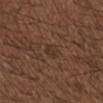Impression: This lesion was catalogued during total-body skin photography and was not selected for biopsy. Image and clinical context: Located on the back. A region of skin cropped from a whole-body photographic capture, roughly 15 mm wide. This is a white-light tile. A male patient, aged approximately 50. Measured at roughly 2.5 mm in maximum diameter. The lesion-visualizer software estimated a lesion color around L≈33 a*≈17 b*≈25 in CIELAB, about 5 CIELAB-L* units darker than the surrounding skin, and a lesion-to-skin contrast of about 5.5 (normalized; higher = more distinct). The analysis additionally found a color-variation rating of about 2/10 and a peripheral color-asymmetry measure near 0.5. And it measured an automated nevus-likeness rating near 0 out of 100.